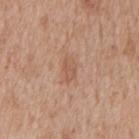Acquisition and patient details: About 3 mm across. A 15 mm close-up extracted from a 3D total-body photography capture. On the mid back. A male subject aged approximately 65.